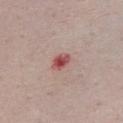Context: A female patient aged 48–52. The total-body-photography lesion software estimated a footprint of about 4 mm² and an outline eccentricity of about 0.75 (0 = round, 1 = elongated). The analysis additionally found a within-lesion color-variation index near 7/10 and a peripheral color-asymmetry measure near 2.5. This is a white-light tile. Approximately 2.5 mm at its widest. A 15 mm crop from a total-body photograph taken for skin-cancer surveillance. Located on the chest.